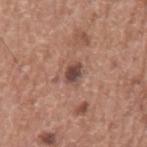biopsy status: catalogued during a skin exam; not biopsied | location: the left upper arm | subject: male, approximately 75 years of age | illumination: white-light | imaging modality: total-body-photography crop, ~15 mm field of view | diameter: ~2.5 mm (longest diameter) | image-analysis metrics: a lesion color around L≈45 a*≈20 b*≈23 in CIELAB, about 13 CIELAB-L* units darker than the surrounding skin, and a lesion-to-skin contrast of about 10 (normalized; higher = more distinct); a lesion-detection confidence of about 100/100.